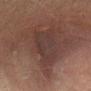Assessment:
This lesion was catalogued during total-body skin photography and was not selected for biopsy.